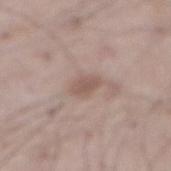imaging modality — ~15 mm crop, total-body skin-cancer survey; patient — male, in their mid- to late 50s; site — the abdomen.